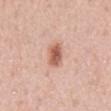| feature | finding |
|---|---|
| biopsy status | imaged on a skin check; not biopsied |
| image-analysis metrics | roughly 13 lightness units darker than nearby skin and a lesion-to-skin contrast of about 8.5 (normalized; higher = more distinct) |
| subject | male, aged 53 to 57 |
| site | the abdomen |
| lesion diameter | ~3.5 mm (longest diameter) |
| imaging modality | ~15 mm tile from a whole-body skin photo |
| tile lighting | white-light |